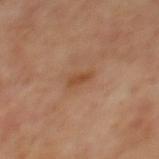The lesion was photographed on a routine skin check and not biopsied; there is no pathology result.